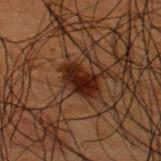<record>
  <biopsy_status>not biopsied; imaged during a skin examination</biopsy_status>
  <image>
    <source>total-body photography crop</source>
    <field_of_view_mm>15</field_of_view_mm>
  </image>
  <automated_metrics>
    <cielab_L>16</cielab_L>
    <cielab_a>17</cielab_a>
    <cielab_b>20</cielab_b>
    <vs_skin_darker_L>11.0</vs_skin_darker_L>
    <vs_skin_contrast_norm>13.0</vs_skin_contrast_norm>
    <color_variation_0_10>3.5</color_variation_0_10>
    <peripheral_color_asymmetry>1.0</peripheral_color_asymmetry>
    <nevus_likeness_0_100>90</nevus_likeness_0_100>
    <lesion_detection_confidence_0_100>100</lesion_detection_confidence_0_100>
  </automated_metrics>
  <patient>
    <sex>male</sex>
    <age_approx>50</age_approx>
  </patient>
  <site>upper back</site>
</record>